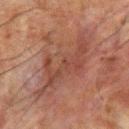Acquisition and patient details: The lesion is located on the back. This image is a 15 mm lesion crop taken from a total-body photograph. Measured at roughly 9.5 mm in maximum diameter. The subject is a male approximately 65 years of age. Imaged with cross-polarized lighting. The lesion-visualizer software estimated a lesion area of about 28 mm² and a symmetry-axis asymmetry near 0.4. And it measured a mean CIELAB color near L≈37 a*≈19 b*≈23 and a normalized lesion–skin contrast near 5.5. The analysis additionally found a lesion-detection confidence of about 75/100.